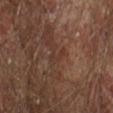Imaged during a routine full-body skin examination; the lesion was not biopsied and no histopathology is available.
On the right forearm.
The tile uses cross-polarized illumination.
The recorded lesion diameter is about 2.5 mm.
A male subject, approximately 65 years of age.
A 15 mm close-up tile from a total-body photography series done for melanoma screening.